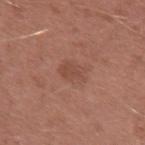<tbp_lesion>
  <biopsy_status>not biopsied; imaged during a skin examination</biopsy_status>
</tbp_lesion>